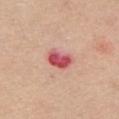  site: chest
  patient:
    sex: female
    age_approx: 65
  lesion_size:
    long_diameter_mm_approx: 3.5
  image:
    source: total-body photography crop
    field_of_view_mm: 15